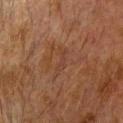Impression:
Imaged during a routine full-body skin examination; the lesion was not biopsied and no histopathology is available.
Background:
Imaged with cross-polarized lighting. From the head or neck. The lesion's longest dimension is about 3.5 mm. The subject is a male about 75 years old. Cropped from a whole-body photographic skin survey; the tile spans about 15 mm.